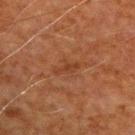• notes — no biopsy performed (imaged during a skin exam)
• image — ~15 mm tile from a whole-body skin photo
• location — the right upper arm
• tile lighting — cross-polarized
• patient — male, aged approximately 70
• lesion size — ≈3 mm
• TBP lesion metrics — an average lesion color of about L≈33 a*≈22 b*≈30 (CIELAB), a lesion–skin lightness drop of about 5, and a normalized lesion–skin contrast near 5.5; a border-irregularity rating of about 5/10 and internal color variation of about 0 on a 0–10 scale